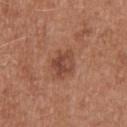Recorded during total-body skin imaging; not selected for excision or biopsy. Longest diameter approximately 3.5 mm. The lesion is on the upper back. Automated tile analysis of the lesion measured a footprint of about 6 mm² and an eccentricity of roughly 0.65. And it measured a lesion color around L≈45 a*≈24 b*≈29 in CIELAB, roughly 9 lightness units darker than nearby skin, and a normalized border contrast of about 7. It also reported a lesion-detection confidence of about 100/100. The tile uses white-light illumination. A 15 mm close-up tile from a total-body photography series done for melanoma screening. A female subject aged 38–42.